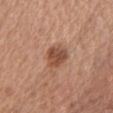The lesion was tiled from a total-body skin photograph and was not biopsied.
Measured at roughly 3.5 mm in maximum diameter.
A female subject, aged 53 to 57.
A lesion tile, about 15 mm wide, cut from a 3D total-body photograph.
On the chest.